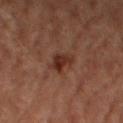  image:
    source: total-body photography crop
    field_of_view_mm: 15
  site: leg
  lighting: cross-polarized
  patient:
    sex: female
    age_approx: 60